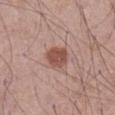Findings:
• follow-up: imaged on a skin check; not biopsied